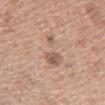• workup · imaged on a skin check; not biopsied
• site · the left upper arm
• acquisition · 15 mm crop, total-body photography
• automated metrics · a lesion area of about 9 mm² and an outline eccentricity of about 0.9 (0 = round, 1 = elongated); a border-irregularity index near 7/10, a within-lesion color-variation index near 4/10, and peripheral color asymmetry of about 1
• patient · female, in their 40s
• lesion diameter · ~5.5 mm (longest diameter)